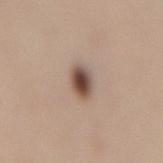Q: Was a biopsy performed?
A: catalogued during a skin exam; not biopsied
Q: What are the patient's age and sex?
A: female, roughly 50 years of age
Q: How large is the lesion?
A: ~3 mm (longest diameter)
Q: What lighting was used for the tile?
A: white-light illumination
Q: What is the anatomic site?
A: the mid back
Q: What is the imaging modality?
A: ~15 mm crop, total-body skin-cancer survey